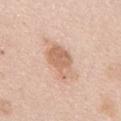Case summary:
– workup · total-body-photography surveillance lesion; no biopsy
– patient · female, aged approximately 65
– body site · the front of the torso
– illumination · white-light
– lesion diameter · ≈4 mm
– image source · ~15 mm tile from a whole-body skin photo